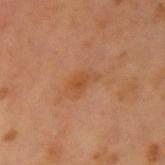Captured during whole-body skin photography for melanoma surveillance; the lesion was not biopsied.
Captured under cross-polarized illumination.
Approximately 3 mm at its widest.
Cropped from a total-body skin-imaging series; the visible field is about 15 mm.
On the left upper arm.
An algorithmic analysis of the crop reported a lesion–skin lightness drop of about 6. It also reported a within-lesion color-variation index near 3/10 and a peripheral color-asymmetry measure near 1.
A male subject in their 60s.